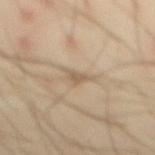Findings:
– notes: catalogued during a skin exam; not biopsied
– lighting: cross-polarized illumination
– lesion diameter: ≈2.5 mm
– anatomic site: the abdomen
– imaging modality: 15 mm crop, total-body photography
– TBP lesion metrics: an area of roughly 2.5 mm², a shape eccentricity near 0.8, and a shape-asymmetry score of about 0.45 (0 = symmetric); a lesion color around L≈57 a*≈13 b*≈29 in CIELAB; internal color variation of about 0.5 on a 0–10 scale and a peripheral color-asymmetry measure near 0; an automated nevus-likeness rating near 0 out of 100 and lesion-presence confidence of about 60/100
– subject: male, about 45 years old